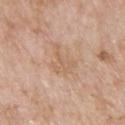Q: Was a biopsy performed?
A: total-body-photography surveillance lesion; no biopsy
Q: What are the patient's age and sex?
A: male, aged around 60
Q: Automated lesion metrics?
A: a lesion area of about 6 mm² and an eccentricity of roughly 0.7; a nevus-likeness score of about 0/100 and a lesion-detection confidence of about 100/100
Q: How large is the lesion?
A: about 4 mm
Q: Lesion location?
A: the upper back
Q: How was this image acquired?
A: ~15 mm crop, total-body skin-cancer survey
Q: How was the tile lit?
A: white-light illumination